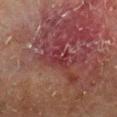The lesion was tiled from a total-body skin photograph and was not biopsied. A male subject, roughly 65 years of age. Located on the left lower leg. A 15 mm crop from a total-body photograph taken for skin-cancer surveillance. The lesion's longest dimension is about 2.5 mm.